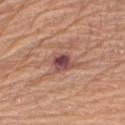A female subject roughly 65 years of age. A close-up tile cropped from a whole-body skin photograph, about 15 mm across. About 2.5 mm across. Located on the right upper arm. This is a white-light tile.